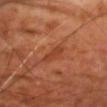– workup · total-body-photography surveillance lesion; no biopsy
– subject · male, in their 70s
– size · ~3 mm (longest diameter)
– lighting · cross-polarized illumination
– anatomic site · the chest
– image-analysis metrics · an area of roughly 2.5 mm² and a symmetry-axis asymmetry near 0.35; an automated nevus-likeness rating near 0 out of 100 and lesion-presence confidence of about 100/100
– imaging modality · total-body-photography crop, ~15 mm field of view